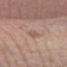Impression:
Imaged during a routine full-body skin examination; the lesion was not biopsied and no histopathology is available.
Acquisition and patient details:
Cropped from a whole-body photographic skin survey; the tile spans about 15 mm. From the arm. Longest diameter approximately 3.5 mm. An algorithmic analysis of the crop reported a footprint of about 2.5 mm² and two-axis asymmetry of about 0.35. The software also gave a mean CIELAB color near L≈56 a*≈18 b*≈24, a lesion–skin lightness drop of about 7, and a normalized border contrast of about 5. And it measured a border-irregularity rating of about 4/10, internal color variation of about 0 on a 0–10 scale, and radial color variation of about 0. This is a white-light tile. A male subject aged 48 to 52.